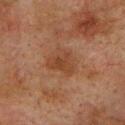Impression: Recorded during total-body skin imaging; not selected for excision or biopsy. Background: About 3.5 mm across. An algorithmic analysis of the crop reported an average lesion color of about L≈35 a*≈19 b*≈28 (CIELAB) and a lesion–skin lightness drop of about 6. The software also gave border irregularity of about 3.5 on a 0–10 scale and a within-lesion color-variation index near 2.5/10. The software also gave a classifier nevus-likeness of about 0/100 and lesion-presence confidence of about 100/100. The subject is a male approximately 75 years of age. On the back. This image is a 15 mm lesion crop taken from a total-body photograph.